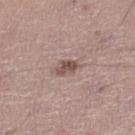biopsy status: catalogued during a skin exam; not biopsied | subject: male, aged 43–47 | site: the left lower leg | size: ~2.5 mm (longest diameter) | image source: total-body-photography crop, ~15 mm field of view.